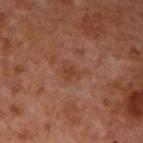From the right upper arm. The lesion's longest dimension is about 2.5 mm. A male patient approximately 55 years of age. This is a cross-polarized tile. Cropped from a total-body skin-imaging series; the visible field is about 15 mm. Automated tile analysis of the lesion measured a footprint of about 3.5 mm², an outline eccentricity of about 0.75 (0 = round, 1 = elongated), and a shape-asymmetry score of about 0.4 (0 = symmetric).